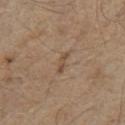Findings:
• follow-up — total-body-photography surveillance lesion; no biopsy
• anatomic site — the chest
• image source — ~15 mm tile from a whole-body skin photo
• lighting — white-light
• subject — male, aged 68 to 72